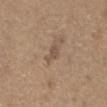Recorded during total-body skin imaging; not selected for excision or biopsy.
The total-body-photography lesion software estimated two-axis asymmetry of about 0.5. The analysis additionally found an average lesion color of about L≈51 a*≈15 b*≈26 (CIELAB) and roughly 8 lightness units darker than nearby skin. It also reported border irregularity of about 4.5 on a 0–10 scale, a within-lesion color-variation index near 1/10, and radial color variation of about 0.
Longest diameter approximately 2.5 mm.
Located on the abdomen.
A male subject, approximately 65 years of age.
The tile uses white-light illumination.
Cropped from a total-body skin-imaging series; the visible field is about 15 mm.